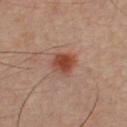This lesion was catalogued during total-body skin photography and was not selected for biopsy. The tile uses cross-polarized illumination. On the front of the torso. A male subject roughly 35 years of age. Cropped from a total-body skin-imaging series; the visible field is about 15 mm. Longest diameter approximately 3 mm.